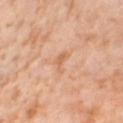Case summary:
– notes: no biopsy performed (imaged during a skin exam)
– image: ~15 mm tile from a whole-body skin photo
– size: about 3 mm
– automated lesion analysis: an average lesion color of about L≈65 a*≈23 b*≈36 (CIELAB) and roughly 7 lightness units darker than nearby skin
– anatomic site: the left thigh
– patient: female, in their mid-50s
– illumination: cross-polarized